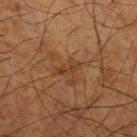No biopsy was performed on this lesion — it was imaged during a full skin examination and was not determined to be concerning.